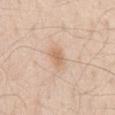{
  "image": {
    "source": "total-body photography crop",
    "field_of_view_mm": 15
  },
  "patient": {
    "sex": "male",
    "age_approx": 45
  },
  "automated_metrics": {
    "area_mm2_approx": 4.5,
    "eccentricity": 0.65,
    "cielab_L": 66,
    "cielab_a": 17,
    "cielab_b": 33,
    "vs_skin_darker_L": 9.0,
    "vs_skin_contrast_norm": 6.0
  },
  "lighting": "white-light",
  "lesion_size": {
    "long_diameter_mm_approx": 2.5
  },
  "site": "lower back"
}